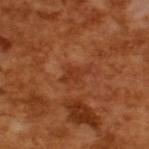workup: no biopsy performed (imaged during a skin exam)
subject: male, in their mid- to late 60s
imaging modality: ~15 mm crop, total-body skin-cancer survey
lesion diameter: about 3 mm
lighting: cross-polarized